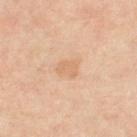An algorithmic analysis of the crop reported an area of roughly 4.5 mm², an outline eccentricity of about 0.55 (0 = round, 1 = elongated), and a symmetry-axis asymmetry near 0.3. It also reported an average lesion color of about L≈70 a*≈20 b*≈36 (CIELAB), roughly 6 lightness units darker than nearby skin, and a lesion-to-skin contrast of about 4.5 (normalized; higher = more distinct).
The lesion's longest dimension is about 2.5 mm.
A region of skin cropped from a whole-body photographic capture, roughly 15 mm wide.
On the right thigh.
The patient is a female aged 48 to 52.
Imaged with cross-polarized lighting.